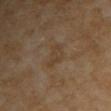No biopsy was performed on this lesion — it was imaged during a full skin examination and was not determined to be concerning.
The lesion is on the arm.
A female patient, aged 68–72.
A close-up tile cropped from a whole-body skin photograph, about 15 mm across.
The lesion-visualizer software estimated a footprint of about 5.5 mm², an outline eccentricity of about 0.8 (0 = round, 1 = elongated), and a symmetry-axis asymmetry near 0.4. It also reported a mean CIELAB color near L≈40 a*≈14 b*≈29 and a lesion-to-skin contrast of about 5 (normalized; higher = more distinct). The software also gave a border-irregularity index near 4.5/10 and internal color variation of about 1.5 on a 0–10 scale.
Captured under cross-polarized illumination.
Approximately 3.5 mm at its widest.